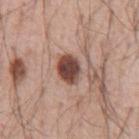On the abdomen.
A 15 mm close-up tile from a total-body photography series done for melanoma screening.
The lesion's longest dimension is about 3.5 mm.
Automated tile analysis of the lesion measured a footprint of about 7.5 mm², an outline eccentricity of about 0.6 (0 = round, 1 = elongated), and two-axis asymmetry of about 0.2. And it measured a mean CIELAB color near L≈45 a*≈21 b*≈24, about 18 CIELAB-L* units darker than the surrounding skin, and a normalized border contrast of about 13.
A male subject aged 53 to 57.
The tile uses white-light illumination.